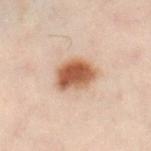Recorded during total-body skin imaging; not selected for excision or biopsy. This is a cross-polarized tile. On the left leg. The subject is a male aged approximately 50. The lesion-visualizer software estimated a lesion color around L≈47 a*≈19 b*≈28 in CIELAB, roughly 14 lightness units darker than nearby skin, and a lesion-to-skin contrast of about 11 (normalized; higher = more distinct). The analysis additionally found border irregularity of about 2 on a 0–10 scale, a color-variation rating of about 4.5/10, and radial color variation of about 1.5. It also reported a classifier nevus-likeness of about 100/100 and a detector confidence of about 100 out of 100 that the crop contains a lesion. This image is a 15 mm lesion crop taken from a total-body photograph. Longest diameter approximately 4.5 mm.